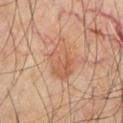Q: Was this lesion biopsied?
A: catalogued during a skin exam; not biopsied
Q: Automated lesion metrics?
A: a footprint of about 11 mm² and a symmetry-axis asymmetry near 0.3; a nevus-likeness score of about 5/100
Q: How was this image acquired?
A: 15 mm crop, total-body photography
Q: Patient demographics?
A: aged approximately 65
Q: Lesion location?
A: the front of the torso